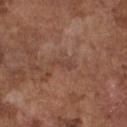workup=total-body-photography surveillance lesion; no biopsy
lesion size=about 2.5 mm
patient=male, in their mid-70s
anatomic site=the chest
acquisition=15 mm crop, total-body photography
TBP lesion metrics=a mean CIELAB color near L≈42 a*≈20 b*≈25, a lesion–skin lightness drop of about 6, and a normalized border contrast of about 5; a border-irregularity rating of about 5.5/10, internal color variation of about 0 on a 0–10 scale, and radial color variation of about 0; a detector confidence of about 100 out of 100 that the crop contains a lesion